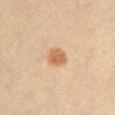anatomic site: the arm; image source: 15 mm crop, total-body photography; lesion diameter: ≈2.5 mm; illumination: cross-polarized; subject: male, roughly 60 years of age.